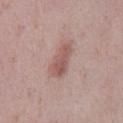Q: Was this lesion biopsied?
A: no biopsy performed (imaged during a skin exam)
Q: What is the imaging modality?
A: 15 mm crop, total-body photography
Q: What are the patient's age and sex?
A: female, in their 40s
Q: What is the anatomic site?
A: the left lower leg
Q: Illumination type?
A: white-light
Q: Automated lesion metrics?
A: a footprint of about 7.5 mm² and two-axis asymmetry of about 0.25; a lesion color around L≈55 a*≈21 b*≈23 in CIELAB, roughly 10 lightness units darker than nearby skin, and a lesion-to-skin contrast of about 7 (normalized; higher = more distinct); a border-irregularity index near 3/10 and a within-lesion color-variation index near 3/10